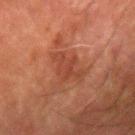follow-up = total-body-photography surveillance lesion; no biopsy
patient = male, aged 78–82
illumination = cross-polarized
location = the left forearm
imaging modality = ~15 mm tile from a whole-body skin photo
diameter = ≈4.5 mm